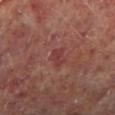{
  "biopsy_status": "not biopsied; imaged during a skin examination",
  "patient": {
    "sex": "male",
    "age_approx": 65
  },
  "site": "left lower leg",
  "image": {
    "source": "total-body photography crop",
    "field_of_view_mm": 15
  }
}